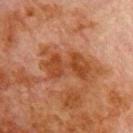Recorded during total-body skin imaging; not selected for excision or biopsy. A roughly 15 mm field-of-view crop from a total-body skin photograph. The tile uses cross-polarized illumination. A male patient about 80 years old. About 6.5 mm across. Automated tile analysis of the lesion measured roughly 8 lightness units darker than nearby skin and a normalized lesion–skin contrast near 8. And it measured a nevus-likeness score of about 0/100 and a lesion-detection confidence of about 100/100. From the front of the torso.